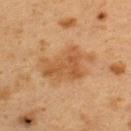Case summary:
* illumination — cross-polarized illumination
* lesion diameter — about 6.5 mm
* patient — female, aged approximately 40
* anatomic site — the upper back
* image source — ~15 mm tile from a whole-body skin photo